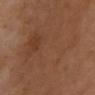Clinical impression:
Captured during whole-body skin photography for melanoma surveillance; the lesion was not biopsied.
Image and clinical context:
Cropped from a total-body skin-imaging series; the visible field is about 15 mm. Captured under cross-polarized illumination. Located on the chest. The patient is a female in their 70s. Measured at roughly 21 mm in maximum diameter.